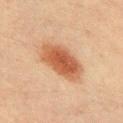{"biopsy_status": "not biopsied; imaged during a skin examination", "lesion_size": {"long_diameter_mm_approx": 6.0}, "patient": {"sex": "male", "age_approx": 35}, "site": "chest", "lighting": "cross-polarized", "image": {"source": "total-body photography crop", "field_of_view_mm": 15}}